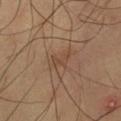notes: catalogued during a skin exam; not biopsied
lighting: cross-polarized illumination
lesion size: ≈3 mm
image: total-body-photography crop, ~15 mm field of view
subject: male, aged 58 to 62
body site: the left thigh